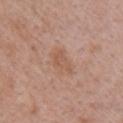Impression:
The lesion was tiled from a total-body skin photograph and was not biopsied.
Background:
A female patient in their 40s. A roughly 15 mm field-of-view crop from a total-body skin photograph. From the right upper arm.